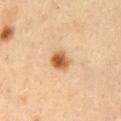Acquisition and patient details:
A 15 mm close-up extracted from a 3D total-body photography capture. The lesion is located on the back. The recorded lesion diameter is about 3 mm. The patient is a female aged 43 to 47.